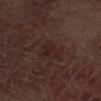The lesion was tiled from a total-body skin photograph and was not biopsied.
Approximately 3 mm at its widest.
A male subject, roughly 70 years of age.
The lesion is on the leg.
Imaged with white-light lighting.
The total-body-photography lesion software estimated an area of roughly 7 mm², a shape eccentricity near 0.55, and two-axis asymmetry of about 0.35. The software also gave a mean CIELAB color near L≈23 a*≈17 b*≈17, about 4 CIELAB-L* units darker than the surrounding skin, and a lesion-to-skin contrast of about 5.5 (normalized; higher = more distinct).
A 15 mm close-up extracted from a 3D total-body photography capture.